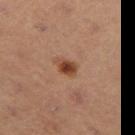– follow-up · no biopsy performed (imaged during a skin exam)
– TBP lesion metrics · a lesion area of about 4 mm²; a mean CIELAB color near L≈42 a*≈22 b*≈31 and a normalized lesion–skin contrast near 10; a within-lesion color-variation index near 4/10 and a peripheral color-asymmetry measure near 1.5
– patient · male, aged approximately 55
– illumination · cross-polarized
– image · total-body-photography crop, ~15 mm field of view
– diameter · ~2.5 mm (longest diameter)
– site · the leg